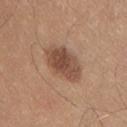The lesion is on the chest. The patient is a male roughly 25 years of age. The tile uses white-light illumination. A region of skin cropped from a whole-body photographic capture, roughly 15 mm wide.